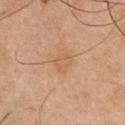<record>
<biopsy_status>not biopsied; imaged during a skin examination</biopsy_status>
<image>
  <source>total-body photography crop</source>
  <field_of_view_mm>15</field_of_view_mm>
</image>
<lighting>cross-polarized</lighting>
<site>chest</site>
<patient>
  <sex>male</sex>
  <age_approx>45</age_approx>
</patient>
<lesion_size>
  <long_diameter_mm_approx>2.5</long_diameter_mm_approx>
</lesion_size>
</record>